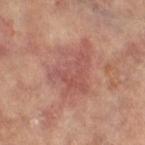biopsy status=total-body-photography surveillance lesion; no biopsy
lighting=cross-polarized
site=the left leg
imaging modality=total-body-photography crop, ~15 mm field of view
size=≈7.5 mm
subject=female, aged 63–67